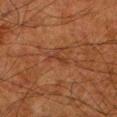Recorded during total-body skin imaging; not selected for excision or biopsy. Automated image analysis of the tile measured a lesion area of about 2.5 mm² and a symmetry-axis asymmetry near 0.65. It also reported a classifier nevus-likeness of about 0/100. This is a cross-polarized tile. This image is a 15 mm lesion crop taken from a total-body photograph. The patient is a male about 80 years old. About 2.5 mm across. The lesion is on the left lower leg.